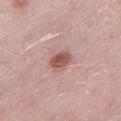Q: Is there a histopathology result?
A: no biopsy performed (imaged during a skin exam)
Q: Automated lesion metrics?
A: a footprint of about 5.5 mm², an outline eccentricity of about 0.6 (0 = round, 1 = elongated), and a symmetry-axis asymmetry near 0.2; an average lesion color of about L≈54 a*≈23 b*≈24 (CIELAB), roughly 13 lightness units darker than nearby skin, and a normalized border contrast of about 8.5; a peripheral color-asymmetry measure near 1; a nevus-likeness score of about 90/100
Q: What is the lesion's diameter?
A: ~3 mm (longest diameter)
Q: Who is the patient?
A: male, approximately 50 years of age
Q: What lighting was used for the tile?
A: white-light
Q: What is the anatomic site?
A: the left lower leg
Q: How was this image acquired?
A: ~15 mm crop, total-body skin-cancer survey